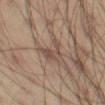Recorded during total-body skin imaging; not selected for excision or biopsy. Cropped from a whole-body photographic skin survey; the tile spans about 15 mm. Located on the left thigh. Longest diameter approximately 3.5 mm. This is a cross-polarized tile. A male patient, aged 38 to 42.